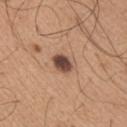Q: What is the lesion's diameter?
A: ≈3 mm
Q: Patient demographics?
A: male, about 65 years old
Q: What is the imaging modality?
A: total-body-photography crop, ~15 mm field of view
Q: Where on the body is the lesion?
A: the right upper arm
Q: Automated lesion metrics?
A: a symmetry-axis asymmetry near 0.2; a border-irregularity index near 1.5/10 and a peripheral color-asymmetry measure near 1.5